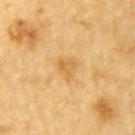Findings:
- notes — total-body-photography surveillance lesion; no biopsy
- anatomic site — the left upper arm
- patient — male, about 85 years old or older
- automated metrics — a mean CIELAB color near L≈55 a*≈17 b*≈42, a lesion–skin lightness drop of about 7, and a normalized lesion–skin contrast near 5.5; an automated nevus-likeness rating near 5 out of 100 and lesion-presence confidence of about 100/100
- diameter — ≈2.5 mm
- acquisition — ~15 mm tile from a whole-body skin photo